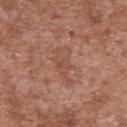Part of a total-body skin-imaging series; this lesion was reviewed on a skin check and was not flagged for biopsy. Captured under white-light illumination. Automated image analysis of the tile measured a lesion area of about 7.5 mm², a shape eccentricity near 0.85, and a shape-asymmetry score of about 0.45 (0 = symmetric). A male subject, roughly 45 years of age. A close-up tile cropped from a whole-body skin photograph, about 15 mm across. On the upper back. About 4.5 mm across.